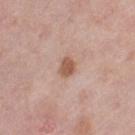workup: imaged on a skin check; not biopsied
illumination: white-light illumination
acquisition: ~15 mm crop, total-body skin-cancer survey
subject: female, approximately 40 years of age
location: the leg